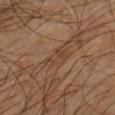| key | value |
|---|---|
| workup | imaged on a skin check; not biopsied |
| body site | the chest |
| automated metrics | a footprint of about 4.5 mm², a shape eccentricity near 0.6, and two-axis asymmetry of about 0.4; a mean CIELAB color near L≈40 a*≈17 b*≈29, about 5 CIELAB-L* units darker than the surrounding skin, and a normalized lesion–skin contrast near 5; a border-irregularity rating of about 3.5/10 and peripheral color asymmetry of about 1.5 |
| tile lighting | cross-polarized illumination |
| imaging modality | ~15 mm crop, total-body skin-cancer survey |
| patient | male, aged 58 to 62 |
| diameter | ≈2.5 mm |